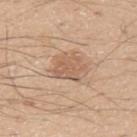No biopsy was performed on this lesion — it was imaged during a full skin examination and was not determined to be concerning. The tile uses white-light illumination. Cropped from a total-body skin-imaging series; the visible field is about 15 mm. The lesion is located on the arm. A male patient, about 30 years old.